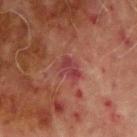Captured during whole-body skin photography for melanoma surveillance; the lesion was not biopsied. On the right upper arm. A close-up tile cropped from a whole-body skin photograph, about 15 mm across. Measured at roughly 3 mm in maximum diameter. The subject is a male aged 68–72.